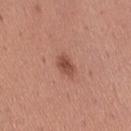Q: Is there a histopathology result?
A: imaged on a skin check; not biopsied